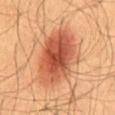{
  "biopsy_status": "not biopsied; imaged during a skin examination",
  "site": "abdomen",
  "patient": {
    "sex": "male",
    "age_approx": 60
  },
  "image": {
    "source": "total-body photography crop",
    "field_of_view_mm": 15
  },
  "lesion_size": {
    "long_diameter_mm_approx": 7.5
  },
  "lighting": "cross-polarized"
}